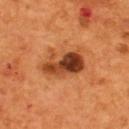Clinical impression: Imaged during a routine full-body skin examination; the lesion was not biopsied and no histopathology is available. Context: A male patient about 55 years old. This image is a 15 mm lesion crop taken from a total-body photograph. The tile uses cross-polarized illumination. The lesion's longest dimension is about 5 mm. Located on the upper back.